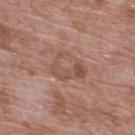notes: total-body-photography surveillance lesion; no biopsy | lighting: white-light | image: ~15 mm tile from a whole-body skin photo | diameter: ≈4 mm | subject: male, approximately 70 years of age | anatomic site: the upper back.